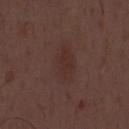Case summary:
- workup · catalogued during a skin exam; not biopsied
- imaging modality · total-body-photography crop, ~15 mm field of view
- subject · male, aged 48 to 52
- TBP lesion metrics · a shape-asymmetry score of about 0.15 (0 = symmetric)
- illumination · white-light
- size · about 5 mm